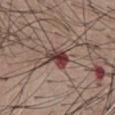Clinical impression:
The lesion was photographed on a routine skin check and not biopsied; there is no pathology result.
Acquisition and patient details:
Captured under white-light illumination. Located on the abdomen. A male subject, in their mid-50s. A lesion tile, about 15 mm wide, cut from a 3D total-body photograph. The total-body-photography lesion software estimated an area of roughly 6.5 mm², a shape eccentricity near 0.8, and two-axis asymmetry of about 0.2. It also reported a mean CIELAB color near L≈41 a*≈21 b*≈20. And it measured a border-irregularity rating of about 2.5/10, a color-variation rating of about 10/10, and radial color variation of about 6. The analysis additionally found a nevus-likeness score of about 0/100 and a lesion-detection confidence of about 100/100. The recorded lesion diameter is about 4 mm.